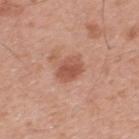The lesion was tiled from a total-body skin photograph and was not biopsied. A male patient about 55 years old. Measured at roughly 3 mm in maximum diameter. From the back. The total-body-photography lesion software estimated a border-irregularity rating of about 1.5/10, internal color variation of about 3 on a 0–10 scale, and a peripheral color-asymmetry measure near 1. It also reported a classifier nevus-likeness of about 65/100 and a detector confidence of about 100 out of 100 that the crop contains a lesion. A lesion tile, about 15 mm wide, cut from a 3D total-body photograph.